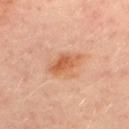Q: Was a biopsy performed?
A: total-body-photography surveillance lesion; no biopsy
Q: What did automated image analysis measure?
A: a footprint of about 9.5 mm², an outline eccentricity of about 0.75 (0 = round, 1 = elongated), and two-axis asymmetry of about 0.25; a lesion–skin lightness drop of about 9 and a normalized lesion–skin contrast near 7; a border-irregularity index near 3/10, a color-variation rating of about 5/10, and radial color variation of about 2
Q: Patient demographics?
A: female, about 50 years old
Q: What is the lesion's diameter?
A: ~4 mm (longest diameter)
Q: What lighting was used for the tile?
A: cross-polarized illumination
Q: What is the imaging modality?
A: ~15 mm crop, total-body skin-cancer survey
Q: What is the anatomic site?
A: the chest